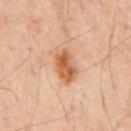follow-up: imaged on a skin check; not biopsied
image source: total-body-photography crop, ~15 mm field of view
illumination: cross-polarized illumination
subject: male, aged 43–47
site: the mid back